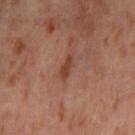Clinical impression: Part of a total-body skin-imaging series; this lesion was reviewed on a skin check and was not flagged for biopsy. Acquisition and patient details: A roughly 15 mm field-of-view crop from a total-body skin photograph. A female subject aged 53 to 57. On the left thigh.